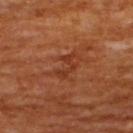Captured during whole-body skin photography for melanoma surveillance; the lesion was not biopsied. Imaged with cross-polarized lighting. Automated image analysis of the tile measured border irregularity of about 8 on a 0–10 scale, a color-variation rating of about 0/10, and a peripheral color-asymmetry measure near 0. A male patient, in their mid-60s. The lesion is on the upper back. Cropped from a whole-body photographic skin survey; the tile spans about 15 mm. Approximately 3.5 mm at its widest.